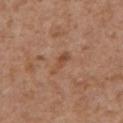About 3 mm across.
The lesion is located on the chest.
A close-up tile cropped from a whole-body skin photograph, about 15 mm across.
A male patient, in their mid-60s.